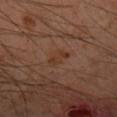Part of a total-body skin-imaging series; this lesion was reviewed on a skin check and was not flagged for biopsy. From the arm. A male patient aged 43 to 47. A close-up tile cropped from a whole-body skin photograph, about 15 mm across.